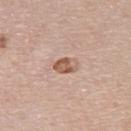Recorded during total-body skin imaging; not selected for excision or biopsy.